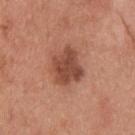No biopsy was performed on this lesion — it was imaged during a full skin examination and was not determined to be concerning. The lesion is located on the back. A 15 mm close-up tile from a total-body photography series done for melanoma screening. The subject is a male about 30 years old.